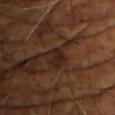Assessment: Captured during whole-body skin photography for melanoma surveillance; the lesion was not biopsied.